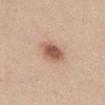Captured during whole-body skin photography for melanoma surveillance; the lesion was not biopsied.
The lesion is on the chest.
Longest diameter approximately 3.5 mm.
A female patient, aged 38 to 42.
The tile uses white-light illumination.
A 15 mm crop from a total-body photograph taken for skin-cancer surveillance.